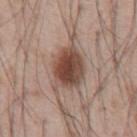Impression: Recorded during total-body skin imaging; not selected for excision or biopsy. Background: A male patient, aged around 55. A lesion tile, about 15 mm wide, cut from a 3D total-body photograph. The lesion is on the abdomen.